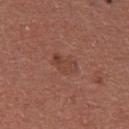Part of a total-body skin-imaging series; this lesion was reviewed on a skin check and was not flagged for biopsy. On the upper back. A region of skin cropped from a whole-body photographic capture, roughly 15 mm wide. About 3 mm across. The lesion-visualizer software estimated an area of roughly 5 mm² and an outline eccentricity of about 0.8 (0 = round, 1 = elongated). And it measured an automated nevus-likeness rating near 0 out of 100. A female patient, aged around 25.